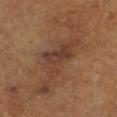Clinical impression:
The lesion was tiled from a total-body skin photograph and was not biopsied.
Image and clinical context:
A female subject, aged approximately 65. Cropped from a total-body skin-imaging series; the visible field is about 15 mm. Located on the right lower leg. Imaged with cross-polarized lighting. The recorded lesion diameter is about 11 mm.